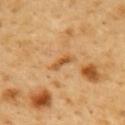• biopsy status: imaged on a skin check; not biopsied
• TBP lesion metrics: an area of roughly 3 mm², a shape eccentricity near 0.9, and a symmetry-axis asymmetry near 0.3; about 10 CIELAB-L* units darker than the surrounding skin and a lesion-to-skin contrast of about 7.5 (normalized; higher = more distinct); internal color variation of about 0 on a 0–10 scale and radial color variation of about 0; a nevus-likeness score of about 0/100 and a detector confidence of about 100 out of 100 that the crop contains a lesion
• illumination: cross-polarized illumination
• imaging modality: 15 mm crop, total-body photography
• patient: male, aged 48 to 52
• site: the back